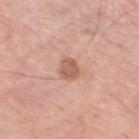A 15 mm crop from a total-body photograph taken for skin-cancer surveillance.
Imaged with white-light lighting.
About 2.5 mm across.
Automated image analysis of the tile measured an area of roughly 5 mm² and a shape eccentricity near 0.65. The analysis additionally found a mean CIELAB color near L≈59 a*≈23 b*≈28 and roughly 11 lightness units darker than nearby skin. The software also gave an automated nevus-likeness rating near 30 out of 100.
The subject is a male in their 80s.
From the leg.